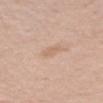  biopsy_status: not biopsied; imaged during a skin examination
  patient:
    sex: male
    age_approx: 70
  image:
    source: total-body photography crop
    field_of_view_mm: 15
  automated_metrics:
    area_mm2_approx: 3.0
    eccentricity: 0.75
    shape_asymmetry: 0.3
    cielab_L: 65
    cielab_a: 17
    cielab_b: 30
    vs_skin_darker_L: 6.0
    vs_skin_contrast_norm: 4.5
    border_irregularity_0_10: 3.0
    color_variation_0_10: 1.0
    peripheral_color_asymmetry: 0.5
    nevus_likeness_0_100: 0
  lesion_size:
    long_diameter_mm_approx: 2.5
  site: right upper arm
  lighting: white-light